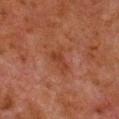  biopsy_status: not biopsied; imaged during a skin examination
  image:
    source: total-body photography crop
    field_of_view_mm: 15
  lighting: cross-polarized
  automated_metrics:
    area_mm2_approx: 5.5
    eccentricity: 0.85
    shape_asymmetry: 0.4
  patient:
    sex: male
    age_approx: 80
  site: right lower leg